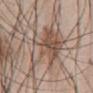• workup: imaged on a skin check; not biopsied
• tile lighting: white-light
• diameter: ≈11.5 mm
• subject: male, about 60 years old
• body site: the front of the torso
• imaging modality: ~15 mm tile from a whole-body skin photo